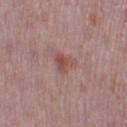No biopsy was performed on this lesion — it was imaged during a full skin examination and was not determined to be concerning.
A close-up tile cropped from a whole-body skin photograph, about 15 mm across.
A female patient approximately 30 years of age.
From the right thigh.